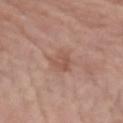This lesion was catalogued during total-body skin photography and was not selected for biopsy. A female patient, aged around 85 or older. Automated tile analysis of the lesion measured a footprint of about 6 mm², an outline eccentricity of about 0.7 (0 = round, 1 = elongated), and a symmetry-axis asymmetry near 0.3. The software also gave a border-irregularity index near 3/10 and a within-lesion color-variation index near 2.5/10. This image is a 15 mm lesion crop taken from a total-body photograph. The lesion is located on the left forearm. Imaged with white-light lighting.